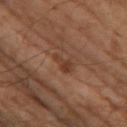Clinical impression: Recorded during total-body skin imaging; not selected for excision or biopsy. Context: The lesion-visualizer software estimated a color-variation rating of about 1.5/10. Imaged with cross-polarized lighting. The patient is a male in their mid- to late 60s. The recorded lesion diameter is about 3 mm. A lesion tile, about 15 mm wide, cut from a 3D total-body photograph.